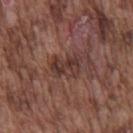notes=total-body-photography surveillance lesion; no biopsy
site=the front of the torso
subject=male, aged approximately 75
size=≈4 mm
imaging modality=~15 mm crop, total-body skin-cancer survey
tile lighting=white-light illumination